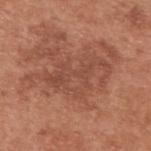A region of skin cropped from a whole-body photographic capture, roughly 15 mm wide. The subject is a male aged 53–57. The tile uses white-light illumination. The lesion is on the upper back.